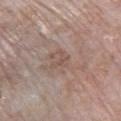Captured during whole-body skin photography for melanoma surveillance; the lesion was not biopsied. A roughly 15 mm field-of-view crop from a total-body skin photograph. An algorithmic analysis of the crop reported a symmetry-axis asymmetry near 0.55. It also reported border irregularity of about 7 on a 0–10 scale, a within-lesion color-variation index near 0/10, and a peripheral color-asymmetry measure near 0. It also reported an automated nevus-likeness rating near 0 out of 100 and a detector confidence of about 100 out of 100 that the crop contains a lesion. The tile uses white-light illumination. A female subject aged approximately 75. The lesion is located on the right lower leg.